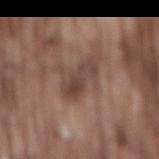Findings:
* follow-up — total-body-photography surveillance lesion; no biopsy
* body site — the mid back
* subject — male, aged around 75
* tile lighting — white-light
* image-analysis metrics — an average lesion color of about L≈42 a*≈17 b*≈22 (CIELAB), roughly 9 lightness units darker than nearby skin, and a lesion-to-skin contrast of about 7.5 (normalized; higher = more distinct)
* imaging modality — total-body-photography crop, ~15 mm field of view
* lesion diameter — ~5 mm (longest diameter)